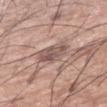Imaged during a routine full-body skin examination; the lesion was not biopsied and no histopathology is available. Located on the left upper arm. A close-up tile cropped from a whole-body skin photograph, about 15 mm across. A male patient aged 53 to 57.